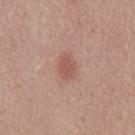<record>
<biopsy_status>not biopsied; imaged during a skin examination</biopsy_status>
<automated_metrics>
  <color_variation_0_10>1.5</color_variation_0_10>
  <peripheral_color_asymmetry>0.5</peripheral_color_asymmetry>
  <lesion_detection_confidence_0_100>100</lesion_detection_confidence_0_100>
</automated_metrics>
<lesion_size>
  <long_diameter_mm_approx>3.0</long_diameter_mm_approx>
</lesion_size>
<patient>
  <sex>male</sex>
  <age_approx>25</age_approx>
</patient>
<image>
  <source>total-body photography crop</source>
  <field_of_view_mm>15</field_of_view_mm>
</image>
<site>chest</site>
<lighting>white-light</lighting>
</record>